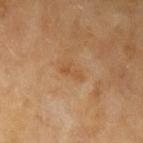Q: Illumination type?
A: cross-polarized illumination
Q: What are the patient's age and sex?
A: female, about 60 years old
Q: How was this image acquired?
A: total-body-photography crop, ~15 mm field of view
Q: What is the anatomic site?
A: the left upper arm
Q: Automated lesion metrics?
A: a border-irregularity rating of about 5.5/10 and a within-lesion color-variation index near 0/10; an automated nevus-likeness rating near 0 out of 100 and a lesion-detection confidence of about 100/100